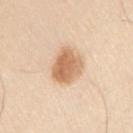The lesion was tiled from a total-body skin photograph and was not biopsied. Imaged with white-light lighting. The lesion's longest dimension is about 4 mm. A 15 mm crop from a total-body photograph taken for skin-cancer surveillance. The lesion is located on the right upper arm. The patient is a male approximately 60 years of age.